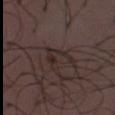This lesion was catalogued during total-body skin photography and was not selected for biopsy.
The lesion is located on the chest.
A roughly 15 mm field-of-view crop from a total-body skin photograph.
The subject is a male aged around 30.